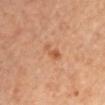Clinical impression: No biopsy was performed on this lesion — it was imaged during a full skin examination and was not determined to be concerning. Acquisition and patient details: A region of skin cropped from a whole-body photographic capture, roughly 15 mm wide. The lesion-visualizer software estimated roughly 8 lightness units darker than nearby skin. The software also gave a nevus-likeness score of about 20/100 and a lesion-detection confidence of about 100/100. The patient is a female roughly 45 years of age. The lesion's longest dimension is about 2.5 mm. This is a cross-polarized tile. On the left upper arm.